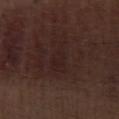Findings:
– workup: total-body-photography surveillance lesion; no biopsy
– patient: male, aged around 70
– imaging modality: 15 mm crop, total-body photography
– lesion size: about 4.5 mm
– site: the right lower leg